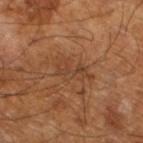Recorded during total-body skin imaging; not selected for excision or biopsy.
A male patient, aged 63–67.
The lesion-visualizer software estimated border irregularity of about 8 on a 0–10 scale and a peripheral color-asymmetry measure near 0.
A roughly 15 mm field-of-view crop from a total-body skin photograph.
The lesion is on the left lower leg.
The recorded lesion diameter is about 4 mm.
Captured under cross-polarized illumination.